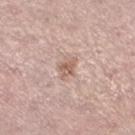No biopsy was performed on this lesion — it was imaged during a full skin examination and was not determined to be concerning.